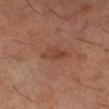image-analysis metrics = a lesion area of about 4.5 mm² and a shape eccentricity near 0.85 | body site = the right lower leg | acquisition = ~15 mm tile from a whole-body skin photo | subject = male, roughly 60 years of age.